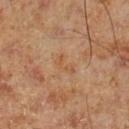Assessment: The lesion was photographed on a routine skin check and not biopsied; there is no pathology result. Context: The patient is a male aged 68–72. A close-up tile cropped from a whole-body skin photograph, about 15 mm across. On the leg. Captured under cross-polarized illumination.